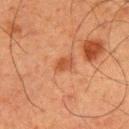The lesion-visualizer software estimated an average lesion color of about L≈41 a*≈24 b*≈32 (CIELAB), about 7 CIELAB-L* units darker than the surrounding skin, and a lesion-to-skin contrast of about 6.5 (normalized; higher = more distinct). And it measured a classifier nevus-likeness of about 65/100 and a lesion-detection confidence of about 100/100. Approximately 2.5 mm at its widest. The subject is a male in their 60s. The lesion is located on the upper back. A 15 mm crop from a total-body photograph taken for skin-cancer surveillance. This is a cross-polarized tile.